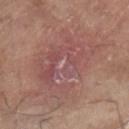Assessment:
Part of a total-body skin-imaging series; this lesion was reviewed on a skin check and was not flagged for biopsy.
Background:
The lesion is located on the right lower leg. The subject is a male roughly 80 years of age. A 15 mm crop from a total-body photograph taken for skin-cancer surveillance.